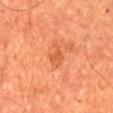The lesion was photographed on a routine skin check and not biopsied; there is no pathology result. A region of skin cropped from a whole-body photographic capture, roughly 15 mm wide. The lesion is located on the chest. About 3 mm across. A male patient, aged 63–67. This is a cross-polarized tile.